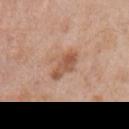• workup — catalogued during a skin exam; not biopsied
• body site — the right upper arm
• patient — male, aged approximately 60
• imaging modality — 15 mm crop, total-body photography